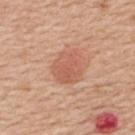Case summary:
- lesion diameter: ≈4 mm
- body site: the back
- lighting: white-light
- patient: female, aged approximately 50
- imaging modality: total-body-photography crop, ~15 mm field of view